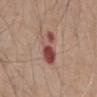Case summary:
- notes: imaged on a skin check; not biopsied
- anatomic site: the abdomen
- image source: ~15 mm crop, total-body skin-cancer survey
- lighting: white-light illumination
- patient: male, aged 78–82